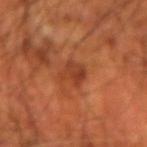Part of a total-body skin-imaging series; this lesion was reviewed on a skin check and was not flagged for biopsy. Approximately 3 mm at its widest. A male patient, aged approximately 70. An algorithmic analysis of the crop reported a lesion area of about 5.5 mm², an outline eccentricity of about 0.55 (0 = round, 1 = elongated), and a symmetry-axis asymmetry near 0.25. From the left forearm. A lesion tile, about 15 mm wide, cut from a 3D total-body photograph.